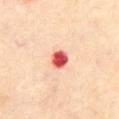notes=no biopsy performed (imaged during a skin exam); location=the chest; patient=male, aged approximately 70; image source=15 mm crop, total-body photography.